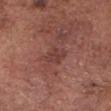Background:
The lesion is on the head or neck. A male patient aged 73 to 77. The tile uses white-light illumination. A 15 mm close-up extracted from a 3D total-body photography capture. The lesion's longest dimension is about 2.5 mm.